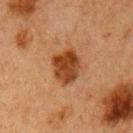notes = catalogued during a skin exam; not biopsied | patient = female, in their 60s | image = 15 mm crop, total-body photography | anatomic site = the left upper arm.